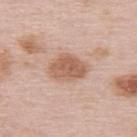The lesion was photographed on a routine skin check and not biopsied; there is no pathology result. The lesion is located on the upper back. Approximately 4.5 mm at its widest. A 15 mm crop from a total-body photograph taken for skin-cancer surveillance. A female subject, aged 63 to 67.